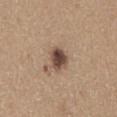Part of a total-body skin-imaging series; this lesion was reviewed on a skin check and was not flagged for biopsy.
The total-body-photography lesion software estimated a footprint of about 6 mm² and a symmetry-axis asymmetry near 0.2. It also reported a lesion color around L≈47 a*≈17 b*≈25 in CIELAB, about 16 CIELAB-L* units darker than the surrounding skin, and a normalized border contrast of about 11.5. It also reported internal color variation of about 5.5 on a 0–10 scale. The analysis additionally found an automated nevus-likeness rating near 75 out of 100 and a detector confidence of about 100 out of 100 that the crop contains a lesion.
Imaged with white-light lighting.
A roughly 15 mm field-of-view crop from a total-body skin photograph.
A male patient aged 63–67.
Approximately 3 mm at its widest.
Located on the front of the torso.